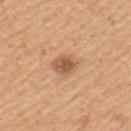Case summary:
- image: total-body-photography crop, ~15 mm field of view
- lesion diameter: about 3.5 mm
- patient: male, approximately 50 years of age
- location: the left upper arm
- automated lesion analysis: an area of roughly 6 mm², a shape eccentricity near 0.75, and two-axis asymmetry of about 0.25; a lesion color around L≈57 a*≈22 b*≈34 in CIELAB, a lesion–skin lightness drop of about 12, and a normalized lesion–skin contrast near 8; a classifier nevus-likeness of about 85/100
- lighting: white-light